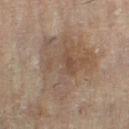Imaged during a routine full-body skin examination; the lesion was not biopsied and no histopathology is available.
Captured under cross-polarized illumination.
The total-body-photography lesion software estimated a lesion area of about 36 mm² and an eccentricity of roughly 0.7. It also reported a lesion–skin lightness drop of about 7 and a lesion-to-skin contrast of about 5.5 (normalized; higher = more distinct). The analysis additionally found a classifier nevus-likeness of about 5/100 and a lesion-detection confidence of about 100/100.
A female subject, in their 80s.
The lesion is on the right leg.
A lesion tile, about 15 mm wide, cut from a 3D total-body photograph.
The recorded lesion diameter is about 9 mm.